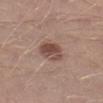Part of a total-body skin-imaging series; this lesion was reviewed on a skin check and was not flagged for biopsy. The lesion-visualizer software estimated a footprint of about 8 mm², an eccentricity of roughly 0.65, and a shape-asymmetry score of about 0.15 (0 = symmetric). It also reported a lesion–skin lightness drop of about 11. A male patient aged 28–32. A lesion tile, about 15 mm wide, cut from a 3D total-body photograph. The tile uses white-light illumination. The lesion is on the right lower leg.